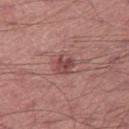This lesion was catalogued during total-body skin photography and was not selected for biopsy. The subject is a male about 45 years old. The lesion is located on the left thigh. Cropped from a whole-body photographic skin survey; the tile spans about 15 mm.